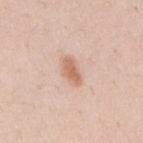The lesion was photographed on a routine skin check and not biopsied; there is no pathology result.
Cropped from a whole-body photographic skin survey; the tile spans about 15 mm.
A male patient, aged 33 to 37.
The tile uses white-light illumination.
On the mid back.
About 3.5 mm across.